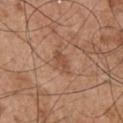Part of a total-body skin-imaging series; this lesion was reviewed on a skin check and was not flagged for biopsy. A 15 mm close-up extracted from a 3D total-body photography capture. The lesion is on the chest. Imaged with white-light lighting. About 3 mm across. The patient is a male aged around 55.